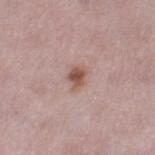No biopsy was performed on this lesion — it was imaged during a full skin examination and was not determined to be concerning.
The tile uses white-light illumination.
A female patient aged approximately 50.
The recorded lesion diameter is about 3 mm.
The lesion is located on the left thigh.
Cropped from a whole-body photographic skin survey; the tile spans about 15 mm.
Automated tile analysis of the lesion measured an outline eccentricity of about 0.65 (0 = round, 1 = elongated) and two-axis asymmetry of about 0.25. The analysis additionally found an automated nevus-likeness rating near 90 out of 100.